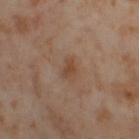Q: Was this lesion biopsied?
A: no biopsy performed (imaged during a skin exam)
Q: Lesion location?
A: the right thigh
Q: How large is the lesion?
A: ≈3 mm
Q: How was this image acquired?
A: ~15 mm crop, total-body skin-cancer survey
Q: Patient demographics?
A: female, aged around 55